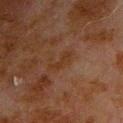The lesion was tiled from a total-body skin photograph and was not biopsied. A roughly 15 mm field-of-view crop from a total-body skin photograph. The subject is a male in their 80s. The lesion is on the chest. Automated tile analysis of the lesion measured peripheral color asymmetry of about 0.